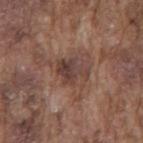{
  "biopsy_status": "not biopsied; imaged during a skin examination",
  "site": "mid back",
  "patient": {
    "sex": "male",
    "age_approx": 75
  },
  "automated_metrics": {
    "area_mm2_approx": 7.0,
    "eccentricity": 0.65,
    "cielab_L": 40,
    "cielab_a": 18,
    "cielab_b": 22,
    "vs_skin_darker_L": 9.0,
    "vs_skin_contrast_norm": 8.5,
    "border_irregularity_0_10": 4.5,
    "color_variation_0_10": 5.0,
    "peripheral_color_asymmetry": 1.5
  },
  "lighting": "white-light",
  "lesion_size": {
    "long_diameter_mm_approx": 3.5
  },
  "image": {
    "source": "total-body photography crop",
    "field_of_view_mm": 15
  }
}